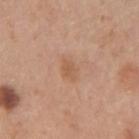The lesion was tiled from a total-body skin photograph and was not biopsied. The lesion is located on the left forearm. Longest diameter approximately 2.5 mm. A female patient, about 35 years old. Automated image analysis of the tile measured border irregularity of about 2.5 on a 0–10 scale, a color-variation rating of about 1.5/10, and a peripheral color-asymmetry measure near 0.5. The analysis additionally found a nevus-likeness score of about 0/100 and lesion-presence confidence of about 100/100. A 15 mm close-up tile from a total-body photography series done for melanoma screening.